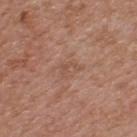| key | value |
|---|---|
| workup | no biopsy performed (imaged during a skin exam) |
| image | 15 mm crop, total-body photography |
| location | the upper back |
| subject | male, aged 53–57 |